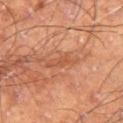The lesion was tiled from a total-body skin photograph and was not biopsied. A 15 mm close-up tile from a total-body photography series done for melanoma screening. A male subject about 60 years old. Located on the leg.